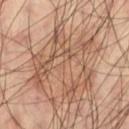Captured during whole-body skin photography for melanoma surveillance; the lesion was not biopsied. A male subject approximately 60 years of age. From the left thigh. A 15 mm close-up tile from a total-body photography series done for melanoma screening. The recorded lesion diameter is about 9 mm.